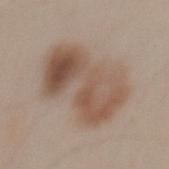Captured during whole-body skin photography for melanoma surveillance; the lesion was not biopsied. A male subject in their 30s. Cropped from a total-body skin-imaging series; the visible field is about 15 mm. Approximately 8.5 mm at its widest. Captured under white-light illumination. Located on the mid back.